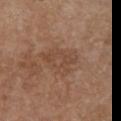{"biopsy_status": "not biopsied; imaged during a skin examination", "site": "front of the torso", "image": {"source": "total-body photography crop", "field_of_view_mm": 15}, "patient": {"sex": "female", "age_approx": 65}, "lighting": "white-light", "automated_metrics": {"area_mm2_approx": 11.0, "eccentricity": 0.65, "cielab_L": 46, "cielab_a": 19, "cielab_b": 29, "vs_skin_darker_L": 6.0, "vs_skin_contrast_norm": 5.0, "border_irregularity_0_10": 3.5, "color_variation_0_10": 3.0, "peripheral_color_asymmetry": 1.0}, "lesion_size": {"long_diameter_mm_approx": 4.5}}